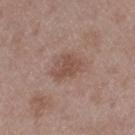Q: What is the lesion's diameter?
A: ≈3 mm
Q: Lesion location?
A: the left thigh
Q: What kind of image is this?
A: ~15 mm tile from a whole-body skin photo
Q: How was the tile lit?
A: white-light
Q: Patient demographics?
A: male, aged 48 to 52